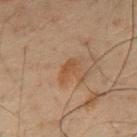No biopsy was performed on this lesion — it was imaged during a full skin examination and was not determined to be concerning.
Imaged with cross-polarized lighting.
The lesion is located on the front of the torso.
Cropped from a total-body skin-imaging series; the visible field is about 15 mm.
A male subject, aged approximately 50.
The lesion-visualizer software estimated an area of roughly 3 mm², an outline eccentricity of about 0.85 (0 = round, 1 = elongated), and a shape-asymmetry score of about 0.3 (0 = symmetric). And it measured an average lesion color of about L≈37 a*≈15 b*≈27 (CIELAB). And it measured a border-irregularity index near 3.5/10 and a peripheral color-asymmetry measure near 0. And it measured a classifier nevus-likeness of about 65/100 and a lesion-detection confidence of about 100/100.
The recorded lesion diameter is about 2.5 mm.